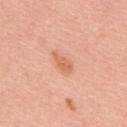This lesion was catalogued during total-body skin photography and was not selected for biopsy.
A close-up tile cropped from a whole-body skin photograph, about 15 mm across.
A male patient about 60 years old.
Located on the back.